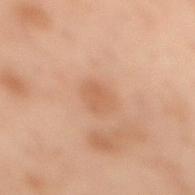notes: imaged on a skin check; not biopsied
acquisition: ~15 mm tile from a whole-body skin photo
patient: female, about 55 years old
body site: the back